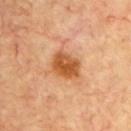  biopsy_status: not biopsied; imaged during a skin examination
  image:
    source: total-body photography crop
    field_of_view_mm: 15
  patient:
    sex: male
    age_approx: 70
  site: chest